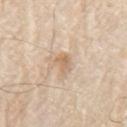Impression: This lesion was catalogued during total-body skin photography and was not selected for biopsy. Background: The lesion's longest dimension is about 3 mm. Located on the left upper arm. A male patient in their 80s. Captured under white-light illumination. A region of skin cropped from a whole-body photographic capture, roughly 15 mm wide.